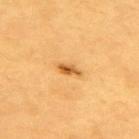No biopsy was performed on this lesion — it was imaged during a full skin examination and was not determined to be concerning. Cropped from a whole-body photographic skin survey; the tile spans about 15 mm. From the upper back. The lesion's longest dimension is about 3 mm. The lesion-visualizer software estimated an average lesion color of about L≈60 a*≈25 b*≈49 (CIELAB), roughly 14 lightness units darker than nearby skin, and a normalized border contrast of about 8.5. The analysis additionally found a border-irregularity index near 3.5/10, internal color variation of about 4.5 on a 0–10 scale, and peripheral color asymmetry of about 1.5. A male patient aged 58–62.